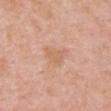follow-up: total-body-photography surveillance lesion; no biopsy | lesion diameter: ≈3 mm | patient: female, aged 68 to 72 | location: the head or neck | image-analysis metrics: an average lesion color of about L≈63 a*≈22 b*≈33 (CIELAB), about 6 CIELAB-L* units darker than the surrounding skin, and a normalized lesion–skin contrast near 5; a classifier nevus-likeness of about 0/100 and a lesion-detection confidence of about 100/100 | tile lighting: white-light illumination | image: total-body-photography crop, ~15 mm field of view.